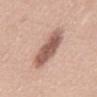Impression: No biopsy was performed on this lesion — it was imaged during a full skin examination and was not determined to be concerning. Background: Approximately 6 mm at its widest. A male patient, aged 48–52. A 15 mm close-up extracted from a 3D total-body photography capture. The tile uses white-light illumination. Automated tile analysis of the lesion measured a footprint of about 15 mm² and an outline eccentricity of about 0.85 (0 = round, 1 = elongated). It also reported a lesion color around L≈57 a*≈21 b*≈25 in CIELAB and about 15 CIELAB-L* units darker than the surrounding skin. The lesion is on the abdomen.